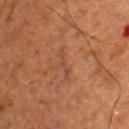{
  "biopsy_status": "not biopsied; imaged during a skin examination",
  "image": {
    "source": "total-body photography crop",
    "field_of_view_mm": 15
  },
  "site": "upper back",
  "patient": {
    "sex": "male",
    "age_approx": 50
  }
}